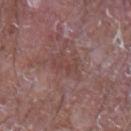Notes:
- notes — no biopsy performed (imaged during a skin exam)
- site — the right upper arm
- automated lesion analysis — a border-irregularity rating of about 4/10 and a color-variation rating of about 1.5/10
- illumination — white-light
- patient — male, aged 63 to 67
- size — ≈3.5 mm
- image — total-body-photography crop, ~15 mm field of view